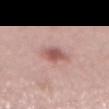notes — catalogued during a skin exam; not biopsied
illumination — white-light illumination
image — 15 mm crop, total-body photography
automated lesion analysis — a lesion area of about 6 mm², a shape eccentricity near 0.65, and two-axis asymmetry of about 0.2; border irregularity of about 2 on a 0–10 scale, a within-lesion color-variation index near 3.5/10, and a peripheral color-asymmetry measure near 1; a nevus-likeness score of about 70/100
body site — the abdomen
subject — female, approximately 40 years of age
size — about 3 mm